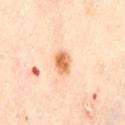The lesion was photographed on a routine skin check and not biopsied; there is no pathology result. On the lower back. A female patient, aged 58 to 62. Measured at roughly 3 mm in maximum diameter. A region of skin cropped from a whole-body photographic capture, roughly 15 mm wide. The lesion-visualizer software estimated an area of roughly 5.5 mm², an eccentricity of roughly 0.65, and two-axis asymmetry of about 0.15. The software also gave a mean CIELAB color near L≈71 a*≈26 b*≈41 and a lesion–skin lightness drop of about 15. And it measured border irregularity of about 1.5 on a 0–10 scale, internal color variation of about 5.5 on a 0–10 scale, and peripheral color asymmetry of about 2. It also reported a classifier nevus-likeness of about 100/100 and lesion-presence confidence of about 100/100. Captured under cross-polarized illumination.